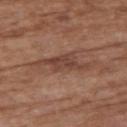The lesion was photographed on a routine skin check and not biopsied; there is no pathology result. The total-body-photography lesion software estimated about 9 CIELAB-L* units darker than the surrounding skin and a normalized lesion–skin contrast near 7. The analysis additionally found a border-irregularity rating of about 6.5/10 and internal color variation of about 3 on a 0–10 scale. The software also gave an automated nevus-likeness rating near 0 out of 100 and a lesion-detection confidence of about 55/100. Measured at roughly 6 mm in maximum diameter. Captured under white-light illumination. A 15 mm close-up extracted from a 3D total-body photography capture. Located on the left upper arm. A male patient, aged around 85 or older.